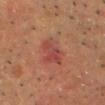notes: catalogued during a skin exam; not biopsied | subject: male, about 60 years old | site: the head or neck | imaging modality: 15 mm crop, total-body photography.